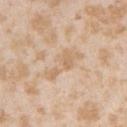notes: imaged on a skin check; not biopsied | body site: the left upper arm | image: total-body-photography crop, ~15 mm field of view | lesion size: about 5 mm | patient: female, aged 23–27 | lighting: white-light illumination.